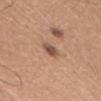{"biopsy_status": "not biopsied; imaged during a skin examination", "image": {"source": "total-body photography crop", "field_of_view_mm": 15}, "site": "head or neck", "patient": {"sex": "female", "age_approx": 40}}